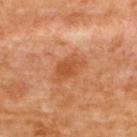Notes:
* follow-up · catalogued during a skin exam; not biopsied
* location · the back
* tile lighting · cross-polarized illumination
* subject · female, about 65 years old
* lesion diameter · ≈3.5 mm
* image source · 15 mm crop, total-body photography
* TBP lesion metrics · an average lesion color of about L≈51 a*≈28 b*≈40 (CIELAB), a lesion–skin lightness drop of about 8, and a lesion-to-skin contrast of about 7 (normalized; higher = more distinct); a border-irregularity rating of about 3/10, a within-lesion color-variation index near 2/10, and a peripheral color-asymmetry measure near 0.5